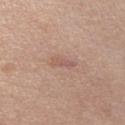Clinical impression: The lesion was tiled from a total-body skin photograph and was not biopsied. Clinical summary: A male subject about 30 years old. Longest diameter approximately 3 mm. Cropped from a total-body skin-imaging series; the visible field is about 15 mm. The total-body-photography lesion software estimated a color-variation rating of about 0/10. And it measured a nevus-likeness score of about 0/100. Imaged with white-light lighting.